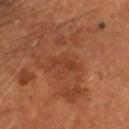notes — no biopsy performed (imaged during a skin exam) | imaging modality — ~15 mm crop, total-body skin-cancer survey | automated lesion analysis — an average lesion color of about L≈37 a*≈25 b*≈32 (CIELAB) and roughly 5 lightness units darker than nearby skin; a classifier nevus-likeness of about 0/100 and lesion-presence confidence of about 100/100 | location — the head or neck | subject — male, in their mid- to late 50s | illumination — cross-polarized.